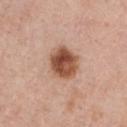follow-up = catalogued during a skin exam; not biopsied | acquisition = total-body-photography crop, ~15 mm field of view | site = the front of the torso | patient = female, approximately 35 years of age | lighting = white-light.